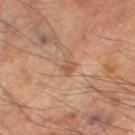This lesion was catalogued during total-body skin photography and was not selected for biopsy.
A male subject aged around 65.
On the right thigh.
The lesion-visualizer software estimated an area of roughly 3 mm², a shape eccentricity near 0.75, and two-axis asymmetry of about 0.3. It also reported roughly 8 lightness units darker than nearby skin and a lesion-to-skin contrast of about 5.5 (normalized; higher = more distinct).
A 15 mm crop from a total-body photograph taken for skin-cancer surveillance.